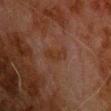| field | value |
|---|---|
| patient | male, about 80 years old |
| lesion size | ~3.5 mm (longest diameter) |
| illumination | cross-polarized illumination |
| image source | total-body-photography crop, ~15 mm field of view |
| location | the chest |